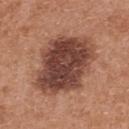Imaged during a routine full-body skin examination; the lesion was not biopsied and no histopathology is available. The recorded lesion diameter is about 8 mm. The lesion is located on the back. A 15 mm close-up tile from a total-body photography series done for melanoma screening. A female subject aged around 40. This is a white-light tile. Automated tile analysis of the lesion measured a nevus-likeness score of about 20/100 and lesion-presence confidence of about 100/100.